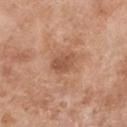{
  "biopsy_status": "not biopsied; imaged during a skin examination",
  "site": "leg",
  "image": {
    "source": "total-body photography crop",
    "field_of_view_mm": 15
  },
  "patient": {
    "sex": "male",
    "age_approx": 80
  },
  "lighting": "white-light",
  "automated_metrics": {
    "border_irregularity_0_10": 2.5,
    "color_variation_0_10": 2.0,
    "peripheral_color_asymmetry": 0.5,
    "nevus_likeness_0_100": 0
  },
  "lesion_size": {
    "long_diameter_mm_approx": 3.0
  }
}